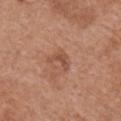The lesion was tiled from a total-body skin photograph and was not biopsied. Cropped from a total-body skin-imaging series; the visible field is about 15 mm. The patient is a female approximately 65 years of age. The lesion is on the chest.